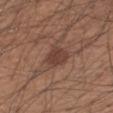This lesion was catalogued during total-body skin photography and was not selected for biopsy. Cropped from a total-body skin-imaging series; the visible field is about 15 mm. About 3.5 mm across. Imaged with white-light lighting. Located on the left forearm. The patient is a male roughly 55 years of age.